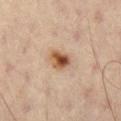biopsy_status: not biopsied; imaged during a skin examination
patient:
  sex: male
  age_approx: 60
lighting: cross-polarized
image:
  source: total-body photography crop
  field_of_view_mm: 15
lesion_size:
  long_diameter_mm_approx: 2.5
site: right thigh